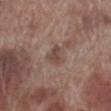Recorded during total-body skin imaging; not selected for excision or biopsy.
The total-body-photography lesion software estimated a footprint of about 3.5 mm², an eccentricity of roughly 0.7, and a shape-asymmetry score of about 0.4 (0 = symmetric). It also reported a border-irregularity index near 4/10 and a within-lesion color-variation index near 1.5/10.
Imaged with white-light lighting.
The patient is a male roughly 70 years of age.
From the right lower leg.
A region of skin cropped from a whole-body photographic capture, roughly 15 mm wide.
The lesion's longest dimension is about 2.5 mm.